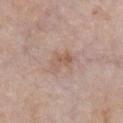Case summary:
– follow-up — total-body-photography surveillance lesion; no biopsy
– location — the chest
– subject — female, approximately 65 years of age
– imaging modality — ~15 mm tile from a whole-body skin photo